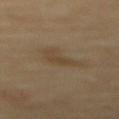Clinical impression:
Recorded during total-body skin imaging; not selected for excision or biopsy.
Acquisition and patient details:
The patient is a male aged 68 to 72. A region of skin cropped from a whole-body photographic capture, roughly 15 mm wide. From the mid back.